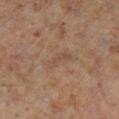Assessment: This lesion was catalogued during total-body skin photography and was not selected for biopsy.